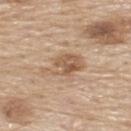Clinical impression: Part of a total-body skin-imaging series; this lesion was reviewed on a skin check and was not flagged for biopsy. Context: This is a white-light tile. The lesion's longest dimension is about 4.5 mm. The lesion is on the upper back. A region of skin cropped from a whole-body photographic capture, roughly 15 mm wide. A male subject, about 80 years old. Automated image analysis of the tile measured a border-irregularity index near 3.5/10 and internal color variation of about 6 on a 0–10 scale.